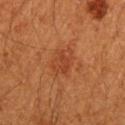{"biopsy_status": "not biopsied; imaged during a skin examination", "site": "left upper arm", "lesion_size": {"long_diameter_mm_approx": 4.0}, "automated_metrics": {"area_mm2_approx": 8.5, "eccentricity": 0.65, "shape_asymmetry": 0.2, "vs_skin_darker_L": 6.0, "vs_skin_contrast_norm": 5.5, "border_irregularity_0_10": 2.0, "color_variation_0_10": 3.0, "peripheral_color_asymmetry": 1.0}, "image": {"source": "total-body photography crop", "field_of_view_mm": 15}, "lighting": "cross-polarized", "patient": {"sex": "male", "age_approx": 60}}